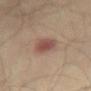Q: Automated lesion metrics?
A: a lesion area of about 7 mm², a shape eccentricity near 0.85, and two-axis asymmetry of about 0.25; a border-irregularity rating of about 2.5/10, a color-variation rating of about 3/10, and radial color variation of about 1
Q: What is the anatomic site?
A: the abdomen
Q: How was the tile lit?
A: cross-polarized illumination
Q: What are the patient's age and sex?
A: male, about 55 years old
Q: What is the imaging modality?
A: total-body-photography crop, ~15 mm field of view